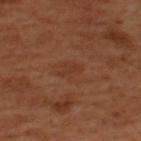Q: Was this lesion biopsied?
A: total-body-photography surveillance lesion; no biopsy
Q: What are the patient's age and sex?
A: male, about 50 years old
Q: Lesion location?
A: the upper back
Q: Lesion size?
A: ≈3 mm
Q: What kind of image is this?
A: ~15 mm tile from a whole-body skin photo
Q: Automated lesion metrics?
A: an average lesion color of about L≈36 a*≈22 b*≈31 (CIELAB) and a normalized border contrast of about 4.5; a border-irregularity rating of about 5/10, a within-lesion color-variation index near 2/10, and radial color variation of about 0.5; a classifier nevus-likeness of about 0/100 and a detector confidence of about 100 out of 100 that the crop contains a lesion
Q: What lighting was used for the tile?
A: cross-polarized illumination